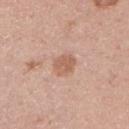This lesion was catalogued during total-body skin photography and was not selected for biopsy.